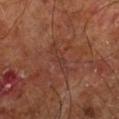| feature | finding |
|---|---|
| workup | no biopsy performed (imaged during a skin exam) |
| tile lighting | cross-polarized illumination |
| subject | male, about 60 years old |
| site | the right leg |
| imaging modality | ~15 mm tile from a whole-body skin photo |
| lesion diameter | ≈5 mm |